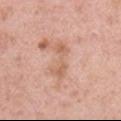Findings:
• biopsy status: total-body-photography surveillance lesion; no biopsy
• patient: male, aged 38–42
• diameter: ~4.5 mm (longest diameter)
• acquisition: ~15 mm tile from a whole-body skin photo
• illumination: white-light illumination
• TBP lesion metrics: a mean CIELAB color near L≈62 a*≈22 b*≈31, a lesion–skin lightness drop of about 8, and a normalized border contrast of about 5.5; a nevus-likeness score of about 0/100 and a lesion-detection confidence of about 100/100
• body site: the right upper arm